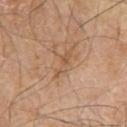– follow-up · total-body-photography surveillance lesion; no biopsy
– TBP lesion metrics · a mean CIELAB color near L≈55 a*≈19 b*≈33, roughly 7 lightness units darker than nearby skin, and a normalized lesion–skin contrast near 5.5
– site · the left upper arm
– patient · male, aged 78 to 82
– tile lighting · white-light
– acquisition · ~15 mm tile from a whole-body skin photo